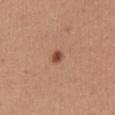Imaged with white-light lighting. The total-body-photography lesion software estimated a mean CIELAB color near L≈48 a*≈24 b*≈31, about 13 CIELAB-L* units darker than the surrounding skin, and a normalized border contrast of about 9. The software also gave a border-irregularity rating of about 1.5/10, a color-variation rating of about 2.5/10, and a peripheral color-asymmetry measure near 0.5. About 2 mm across. A female patient approximately 45 years of age. Located on the chest. A close-up tile cropped from a whole-body skin photograph, about 15 mm across.